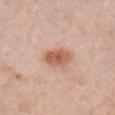| feature | finding |
|---|---|
| notes | catalogued during a skin exam; not biopsied |
| acquisition | 15 mm crop, total-body photography |
| illumination | white-light |
| body site | the chest |
| image-analysis metrics | a footprint of about 8 mm², an outline eccentricity of about 0.8 (0 = round, 1 = elongated), and two-axis asymmetry of about 0.2; an average lesion color of about L≈59 a*≈24 b*≈31 (CIELAB), roughly 12 lightness units darker than nearby skin, and a lesion-to-skin contrast of about 8.5 (normalized; higher = more distinct); an automated nevus-likeness rating near 95 out of 100 and a lesion-detection confidence of about 100/100 |
| size | ≈4 mm |
| subject | female, aged around 50 |